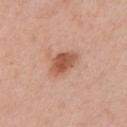Assessment: No biopsy was performed on this lesion — it was imaged during a full skin examination and was not determined to be concerning. Background: Captured under white-light illumination. The total-body-photography lesion software estimated a mean CIELAB color near L≈55 a*≈25 b*≈31 and a lesion-to-skin contrast of about 8.5 (normalized; higher = more distinct). It also reported border irregularity of about 2 on a 0–10 scale, a within-lesion color-variation index near 4/10, and a peripheral color-asymmetry measure near 1.5. The analysis additionally found a classifier nevus-likeness of about 95/100 and a lesion-detection confidence of about 100/100. Cropped from a total-body skin-imaging series; the visible field is about 15 mm. A female patient, approximately 40 years of age. The lesion is located on the chest.